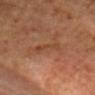Part of a total-body skin-imaging series; this lesion was reviewed on a skin check and was not flagged for biopsy. The lesion is on the head or neck. Imaged with cross-polarized lighting. A male patient approximately 70 years of age. A region of skin cropped from a whole-body photographic capture, roughly 15 mm wide. The recorded lesion diameter is about 4 mm. The lesion-visualizer software estimated a lesion color around L≈44 a*≈22 b*≈34 in CIELAB, a lesion–skin lightness drop of about 6, and a normalized lesion–skin contrast near 5.5. The analysis additionally found a lesion-detection confidence of about 95/100.